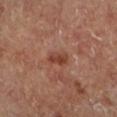The lesion was tiled from a total-body skin photograph and was not biopsied.
The lesion's longest dimension is about 2.5 mm.
A close-up tile cropped from a whole-body skin photograph, about 15 mm across.
On the left lower leg.
The lesion-visualizer software estimated a lesion–skin lightness drop of about 9 and a lesion-to-skin contrast of about 8 (normalized; higher = more distinct). The software also gave an automated nevus-likeness rating near 5 out of 100 and lesion-presence confidence of about 100/100.
A male subject, aged 63–67.
This is a cross-polarized tile.